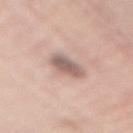Longest diameter approximately 4.5 mm. This image is a 15 mm lesion crop taken from a total-body photograph. A female subject, roughly 60 years of age. This is a white-light tile. The lesion is on the mid back.